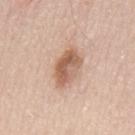Findings:
• follow-up · no biopsy performed (imaged during a skin exam)
• imaging modality · 15 mm crop, total-body photography
• subject · male, in their mid-60s
• site · the mid back